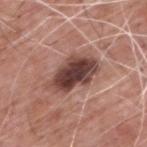Part of a total-body skin-imaging series; this lesion was reviewed on a skin check and was not flagged for biopsy.
A roughly 15 mm field-of-view crop from a total-body skin photograph.
A male patient, about 60 years old.
Located on the upper back.